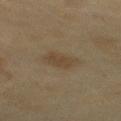notes = no biopsy performed (imaged during a skin exam); patient = female, roughly 60 years of age; image source = total-body-photography crop, ~15 mm field of view; location = the upper back; lighting = cross-polarized illumination; lesion size = ≈3.5 mm; TBP lesion metrics = a lesion–skin lightness drop of about 6 and a normalized lesion–skin contrast near 5.5.